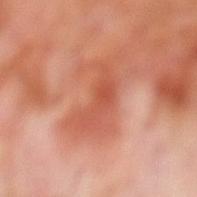The lesion was photographed on a routine skin check and not biopsied; there is no pathology result. About 6 mm across. On the left lower leg. A male subject, about 70 years old. A 15 mm close-up extracted from a 3D total-body photography capture.